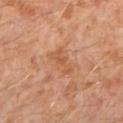| field | value |
|---|---|
| biopsy status | catalogued during a skin exam; not biopsied |
| subject | male, approximately 30 years of age |
| location | the left lower leg |
| image | ~15 mm crop, total-body skin-cancer survey |